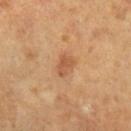tile lighting: cross-polarized illumination | diameter: about 2.5 mm | patient: female, aged around 65 | body site: the left forearm | acquisition: total-body-photography crop, ~15 mm field of view.